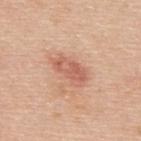Clinical impression:
This lesion was catalogued during total-body skin photography and was not selected for biopsy.
Clinical summary:
About 4.5 mm across. Cropped from a whole-body photographic skin survey; the tile spans about 15 mm. A male patient, aged 48–52. Automated tile analysis of the lesion measured an outline eccentricity of about 0.85 (0 = round, 1 = elongated) and a shape-asymmetry score of about 0.25 (0 = symmetric). And it measured an average lesion color of about L≈60 a*≈25 b*≈31 (CIELAB), roughly 10 lightness units darker than nearby skin, and a normalized border contrast of about 6.5. The analysis additionally found border irregularity of about 3 on a 0–10 scale, internal color variation of about 3.5 on a 0–10 scale, and peripheral color asymmetry of about 1.5. The software also gave an automated nevus-likeness rating near 70 out of 100. The lesion is located on the upper back. The tile uses white-light illumination.